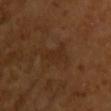Q: Was this lesion biopsied?
A: catalogued during a skin exam; not biopsied
Q: Who is the patient?
A: female, roughly 55 years of age
Q: Lesion size?
A: ~4 mm (longest diameter)
Q: Lesion location?
A: the right upper arm
Q: What lighting was used for the tile?
A: cross-polarized
Q: Automated lesion metrics?
A: an eccentricity of roughly 0.85 and a symmetry-axis asymmetry near 0.45; about 4 CIELAB-L* units darker than the surrounding skin; a border-irregularity rating of about 5/10, a color-variation rating of about 2/10, and radial color variation of about 0.5; an automated nevus-likeness rating near 0 out of 100 and a lesion-detection confidence of about 100/100
Q: How was this image acquired?
A: total-body-photography crop, ~15 mm field of view